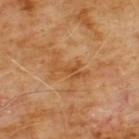Findings:
* biopsy status — catalogued during a skin exam; not biopsied
* acquisition — total-body-photography crop, ~15 mm field of view
* site — the front of the torso
* subject — male, approximately 60 years of age